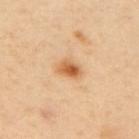Case summary:
* biopsy status · total-body-photography surveillance lesion; no biopsy
* TBP lesion metrics · an automated nevus-likeness rating near 95 out of 100 and a detector confidence of about 100 out of 100 that the crop contains a lesion
* imaging modality · ~15 mm tile from a whole-body skin photo
* patient · female, approximately 40 years of age
* body site · the upper back
* lesion size · ~3 mm (longest diameter)